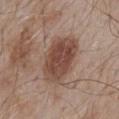• workup — total-body-photography surveillance lesion; no biopsy
• illumination — white-light
• lesion size — about 6.5 mm
• image source — 15 mm crop, total-body photography
• anatomic site — the back
• patient — male, aged 53–57
• image-analysis metrics — a lesion area of about 19 mm² and a symmetry-axis asymmetry near 0.2; a nevus-likeness score of about 80/100